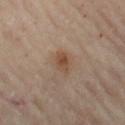No biopsy was performed on this lesion — it was imaged during a full skin examination and was not determined to be concerning. A female patient about 60 years old. Approximately 2.5 mm at its widest. The lesion is located on the left thigh. Cropped from a whole-body photographic skin survey; the tile spans about 15 mm. This is a cross-polarized tile. Automated image analysis of the tile measured an area of roughly 4 mm² and a symmetry-axis asymmetry near 0.25. And it measured a lesion color around L≈47 a*≈17 b*≈29 in CIELAB, roughly 8 lightness units darker than nearby skin, and a normalized lesion–skin contrast near 7. And it measured a border-irregularity index near 2/10, internal color variation of about 4.5 on a 0–10 scale, and peripheral color asymmetry of about 1.5. The software also gave a nevus-likeness score of about 55/100.